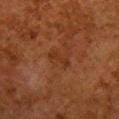The lesion is located on the chest. The patient is a female aged approximately 50. Cropped from a whole-body photographic skin survey; the tile spans about 15 mm.